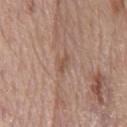{"biopsy_status": "not biopsied; imaged during a skin examination", "image": {"source": "total-body photography crop", "field_of_view_mm": 15}, "automated_metrics": {"cielab_L": 52, "cielab_a": 19, "cielab_b": 28, "vs_skin_darker_L": 8.0, "vs_skin_contrast_norm": 6.0, "color_variation_0_10": 0.0, "peripheral_color_asymmetry": 0.0, "nevus_likeness_0_100": 0, "lesion_detection_confidence_0_100": 95}, "patient": {"sex": "male", "age_approx": 65}, "site": "chest", "lesion_size": {"long_diameter_mm_approx": 2.5}}